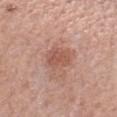Clinical impression:
Captured during whole-body skin photography for melanoma surveillance; the lesion was not biopsied.
Clinical summary:
A 15 mm close-up extracted from a 3D total-body photography capture. The lesion is located on the left forearm. The patient is a female aged around 60.